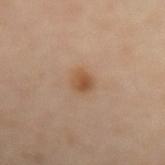This lesion was catalogued during total-body skin photography and was not selected for biopsy. On the left forearm. The tile uses cross-polarized illumination. A 15 mm crop from a total-body photograph taken for skin-cancer surveillance. Automated image analysis of the tile measured an area of roughly 4 mm². The analysis additionally found a lesion color around L≈52 a*≈19 b*≈34 in CIELAB, roughly 9 lightness units darker than nearby skin, and a lesion-to-skin contrast of about 8 (normalized; higher = more distinct). Measured at roughly 2.5 mm in maximum diameter. The patient is a female in their mid-40s.